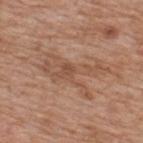This lesion was catalogued during total-body skin photography and was not selected for biopsy. Cropped from a total-body skin-imaging series; the visible field is about 15 mm. From the upper back. The subject is a male aged 58–62. This is a white-light tile. Approximately 7.5 mm at its widest.